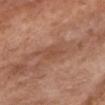No biopsy was performed on this lesion — it was imaged during a full skin examination and was not determined to be concerning. A 15 mm crop from a total-body photograph taken for skin-cancer surveillance. The lesion is located on the chest. Imaged with white-light lighting. The patient is a female about 75 years old. The total-body-photography lesion software estimated an area of roughly 5 mm² and a shape-asymmetry score of about 0.2 (0 = symmetric).